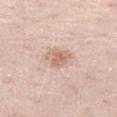{"biopsy_status": "not biopsied; imaged during a skin examination", "lesion_size": {"long_diameter_mm_approx": 3.0}, "automated_metrics": {"area_mm2_approx": 6.0, "eccentricity": 0.5, "lesion_detection_confidence_0_100": 100}, "patient": {"sex": "male", "age_approx": 50}, "image": {"source": "total-body photography crop", "field_of_view_mm": 15}, "lighting": "white-light", "site": "left thigh"}